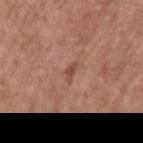Acquisition and patient details:
A roughly 15 mm field-of-view crop from a total-body skin photograph. Captured under white-light illumination. Automated image analysis of the tile measured an average lesion color of about L≈48 a*≈21 b*≈28 (CIELAB), about 9 CIELAB-L* units darker than the surrounding skin, and a normalized border contrast of about 6.5. It also reported a border-irregularity rating of about 4/10, internal color variation of about 0 on a 0–10 scale, and peripheral color asymmetry of about 0. The lesion is on the left upper arm. The lesion's longest dimension is about 2.5 mm. A male patient about 75 years old.